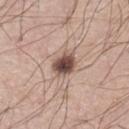This lesion was catalogued during total-body skin photography and was not selected for biopsy. A male subject, aged 38–42. An algorithmic analysis of the crop reported a border-irregularity rating of about 2/10, a color-variation rating of about 5/10, and peripheral color asymmetry of about 1.5. The software also gave a nevus-likeness score of about 95/100 and a lesion-detection confidence of about 100/100. The lesion is located on the left lower leg. A 15 mm close-up tile from a total-body photography series done for melanoma screening. Captured under white-light illumination. Approximately 3 mm at its widest.